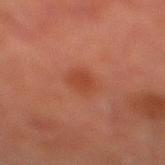<record>
<biopsy_status>not biopsied; imaged during a skin examination</biopsy_status>
<lighting>cross-polarized</lighting>
<automated_metrics>
  <border_irregularity_0_10>2.0</border_irregularity_0_10>
  <color_variation_0_10>1.5</color_variation_0_10>
</automated_metrics>
<site>left lower leg</site>
<image>
  <source>total-body photography crop</source>
  <field_of_view_mm>15</field_of_view_mm>
</image>
<patient>
  <sex>male</sex>
  <age_approx>70</age_approx>
</patient>
</record>